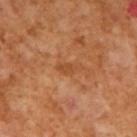Q: Is there a histopathology result?
A: catalogued during a skin exam; not biopsied
Q: Lesion size?
A: ~3 mm (longest diameter)
Q: What kind of image is this?
A: ~15 mm tile from a whole-body skin photo
Q: What did automated image analysis measure?
A: a border-irregularity index near 4/10, a within-lesion color-variation index near 0.5/10, and radial color variation of about 0.5
Q: What are the patient's age and sex?
A: male, in their mid- to late 60s
Q: Illumination type?
A: cross-polarized